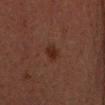Notes:
* follow-up — no biopsy performed (imaged during a skin exam)
* image source — ~15 mm tile from a whole-body skin photo
* size — about 2.5 mm
* patient — male, aged approximately 30
* lighting — cross-polarized illumination
* location — the head or neck